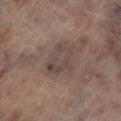- patient: male, aged approximately 65
- body site: the left lower leg
- tile lighting: cross-polarized illumination
- automated metrics: an area of roughly 9.5 mm² and an eccentricity of roughly 0.65; a border-irregularity index near 3.5/10 and internal color variation of about 5.5 on a 0–10 scale
- diameter: about 4 mm
- imaging modality: ~15 mm crop, total-body skin-cancer survey